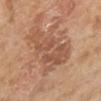Notes:
– biopsy status: catalogued during a skin exam; not biopsied
– subject: male, aged 63–67
– location: the right lower leg
– image: ~15 mm tile from a whole-body skin photo
– lesion size: ≈8 mm
– tile lighting: cross-polarized